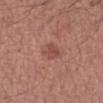- biopsy status: total-body-photography surveillance lesion; no biopsy
- automated metrics: an average lesion color of about L≈48 a*≈24 b*≈27 (CIELAB), a lesion–skin lightness drop of about 8, and a normalized lesion–skin contrast near 6
- body site: the left forearm
- lighting: white-light
- lesion size: ≈2.5 mm
- image source: ~15 mm tile from a whole-body skin photo
- subject: male, aged 53–57